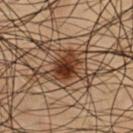Captured during whole-body skin photography for melanoma surveillance; the lesion was not biopsied.
The subject is a male in their mid-50s.
Cropped from a whole-body photographic skin survey; the tile spans about 15 mm.
From the chest.
Captured under cross-polarized illumination.
Longest diameter approximately 3.5 mm.